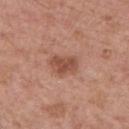  biopsy_status: not biopsied; imaged during a skin examination
  image:
    source: total-body photography crop
    field_of_view_mm: 15
  lesion_size:
    long_diameter_mm_approx: 3.5
  automated_metrics:
    area_mm2_approx: 7.0
    eccentricity: 0.75
    shape_asymmetry: 0.3
    vs_skin_darker_L: 10.0
    vs_skin_contrast_norm: 7.5
    border_irregularity_0_10: 2.5
    color_variation_0_10: 2.5
    peripheral_color_asymmetry: 1.0
    nevus_likeness_0_100: 85
    lesion_detection_confidence_0_100: 100
  site: upper back
  patient:
    sex: male
    age_approx: 65